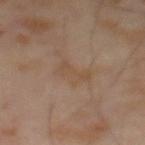Part of a total-body skin-imaging series; this lesion was reviewed on a skin check and was not flagged for biopsy. A male patient, about 65 years old. Cropped from a total-body skin-imaging series; the visible field is about 15 mm. Captured under cross-polarized illumination.